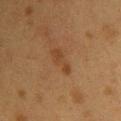| key | value |
|---|---|
| follow-up | catalogued during a skin exam; not biopsied |
| image source | ~15 mm tile from a whole-body skin photo |
| patient | female, aged 38 to 42 |
| size | about 3.5 mm |
| lighting | cross-polarized |
| anatomic site | the back |
| TBP lesion metrics | a footprint of about 4.5 mm², a shape eccentricity near 0.9, and a symmetry-axis asymmetry near 0.35; roughly 6 lightness units darker than nearby skin and a normalized border contrast of about 6; a border-irregularity rating of about 4.5/10, internal color variation of about 1 on a 0–10 scale, and peripheral color asymmetry of about 0 |